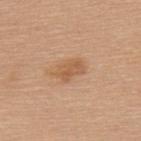Case summary:
- biopsy status — imaged on a skin check; not biopsied
- tile lighting — white-light
- diameter — ≈3.5 mm
- subject — female, about 60 years old
- location — the back
- image source — total-body-photography crop, ~15 mm field of view
- image-analysis metrics — a border-irregularity rating of about 2.5/10 and internal color variation of about 2.5 on a 0–10 scale; lesion-presence confidence of about 100/100